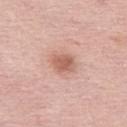Part of a total-body skin-imaging series; this lesion was reviewed on a skin check and was not flagged for biopsy. Measured at roughly 3 mm in maximum diameter. Imaged with white-light lighting. Automated tile analysis of the lesion measured a border-irregularity index near 2/10, a within-lesion color-variation index near 3/10, and peripheral color asymmetry of about 1. And it measured a nevus-likeness score of about 80/100 and lesion-presence confidence of about 100/100. Cropped from a total-body skin-imaging series; the visible field is about 15 mm. The lesion is on the left thigh. The patient is a female approximately 65 years of age.